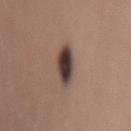- workup · catalogued during a skin exam; not biopsied
- patient · female, in their mid-50s
- location · the lower back
- image source · ~15 mm tile from a whole-body skin photo